Located on the arm · a male patient, about 45 years old · this image is a 15 mm lesion crop taken from a total-body photograph: 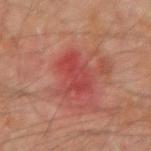Conclusion: Histopathologically confirmed as a superficial basal cell carcinoma (malignant).